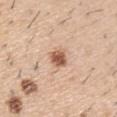Clinical impression: Recorded during total-body skin imaging; not selected for excision or biopsy. Acquisition and patient details: The lesion is located on the right upper arm. Cropped from a total-body skin-imaging series; the visible field is about 15 mm. The patient is a male roughly 60 years of age. The lesion's longest dimension is about 2.5 mm. Imaged with white-light lighting.